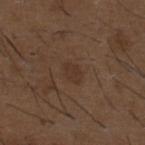notes = catalogued during a skin exam; not biopsied
automated lesion analysis = a nevus-likeness score of about 0/100 and a lesion-detection confidence of about 100/100
diameter = ~2.5 mm (longest diameter)
tile lighting = white-light
patient = male, in their 50s
imaging modality = ~15 mm crop, total-body skin-cancer survey
anatomic site = the upper back